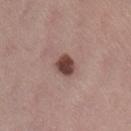Assessment: Part of a total-body skin-imaging series; this lesion was reviewed on a skin check and was not flagged for biopsy. Background: A lesion tile, about 15 mm wide, cut from a 3D total-body photograph. Captured under white-light illumination. A female patient aged 48 to 52. The lesion is located on the back. Measured at roughly 2.5 mm in maximum diameter.